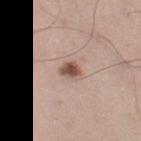Imaged during a routine full-body skin examination; the lesion was not biopsied and no histopathology is available.
A male subject aged 38 to 42.
A 15 mm close-up tile from a total-body photography series done for melanoma screening.
Located on the left thigh.
Captured under white-light illumination.